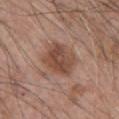Part of a total-body skin-imaging series; this lesion was reviewed on a skin check and was not flagged for biopsy. The subject is a male roughly 70 years of age. Automated image analysis of the tile measured about 10 CIELAB-L* units darker than the surrounding skin and a normalized lesion–skin contrast near 8. The software also gave an automated nevus-likeness rating near 35 out of 100 and a detector confidence of about 100 out of 100 that the crop contains a lesion. On the front of the torso. This image is a 15 mm lesion crop taken from a total-body photograph. The lesion's longest dimension is about 4.5 mm.